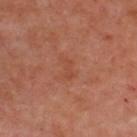Imaged during a routine full-body skin examination; the lesion was not biopsied and no histopathology is available.
A 15 mm close-up tile from a total-body photography series done for melanoma screening.
The tile uses cross-polarized illumination.
The lesion is located on the upper back.
A male patient, aged 48 to 52.
Longest diameter approximately 2.5 mm.